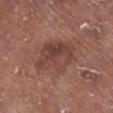Case summary:
– biopsy status: no biopsy performed (imaged during a skin exam)
– image: total-body-photography crop, ~15 mm field of view
– lesion diameter: about 6 mm
– patient: male, roughly 75 years of age
– site: the right lower leg
– lighting: white-light